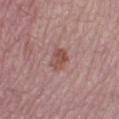Captured during whole-body skin photography for melanoma surveillance; the lesion was not biopsied. The lesion is on the right lower leg. A 15 mm close-up extracted from a 3D total-body photography capture. The total-body-photography lesion software estimated a lesion area of about 5 mm², an eccentricity of roughly 0.65, and a shape-asymmetry score of about 0.15 (0 = symmetric). The software also gave border irregularity of about 2 on a 0–10 scale, a color-variation rating of about 3/10, and a peripheral color-asymmetry measure near 1. And it measured an automated nevus-likeness rating near 45 out of 100 and lesion-presence confidence of about 100/100. Imaged with white-light lighting. A female patient about 70 years old. The lesion's longest dimension is about 3 mm.